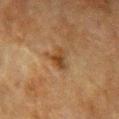Notes:
– workup · total-body-photography surveillance lesion; no biopsy
– image source · 15 mm crop, total-body photography
– subject · female, about 55 years old
– tile lighting · cross-polarized
– diameter · ≈3 mm
– image-analysis metrics · a border-irregularity index near 4/10 and a peripheral color-asymmetry measure near 1
– site · the chest